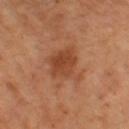| feature | finding |
|---|---|
| follow-up | no biopsy performed (imaged during a skin exam) |
| patient | female, approximately 55 years of age |
| acquisition | ~15 mm tile from a whole-body skin photo |
| location | the left thigh |